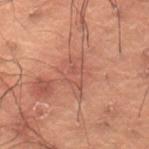No biopsy was performed on this lesion — it was imaged during a full skin examination and was not determined to be concerning.
A male patient, aged approximately 50.
From the lower back.
A 15 mm close-up tile from a total-body photography series done for melanoma screening.
Captured under cross-polarized illumination.